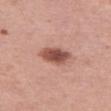workup = no biopsy performed (imaged during a skin exam) | imaging modality = ~15 mm crop, total-body skin-cancer survey | subject = female, aged approximately 40 | lesion size = about 3.5 mm | body site = the left thigh.